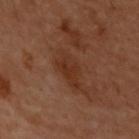<tbp_lesion>
<biopsy_status>not biopsied; imaged during a skin examination</biopsy_status>
<site>upper back</site>
<lighting>cross-polarized</lighting>
<image>
  <source>total-body photography crop</source>
  <field_of_view_mm>15</field_of_view_mm>
</image>
<patient>
  <sex>female</sex>
  <age_approx>60</age_approx>
</patient>
<automated_metrics>
  <area_mm2_approx>7.0</area_mm2_approx>
  <eccentricity>0.8</eccentricity>
  <cielab_L>27</cielab_L>
  <cielab_a>19</cielab_a>
  <cielab_b>26</cielab_b>
  <vs_skin_darker_L>6.0</vs_skin_darker_L>
  <nevus_likeness_0_100>0</nevus_likeness_0_100>
</automated_metrics>
<lesion_size>
  <long_diameter_mm_approx>4.0</long_diameter_mm_approx>
</lesion_size>
</tbp_lesion>